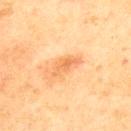Captured during whole-body skin photography for melanoma surveillance; the lesion was not biopsied. Located on the upper back. The lesion's longest dimension is about 4 mm. A 15 mm crop from a total-body photograph taken for skin-cancer surveillance. This is a cross-polarized tile. A male patient, aged around 45.